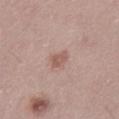This lesion was catalogued during total-body skin photography and was not selected for biopsy.
The lesion-visualizer software estimated a footprint of about 3 mm², an outline eccentricity of about 0.8 (0 = round, 1 = elongated), and a shape-asymmetry score of about 0.3 (0 = symmetric). And it measured border irregularity of about 2.5 on a 0–10 scale.
The tile uses white-light illumination.
A 15 mm crop from a total-body photograph taken for skin-cancer surveillance.
The lesion is on the left thigh.
The recorded lesion diameter is about 2.5 mm.
The patient is a female aged 28 to 32.